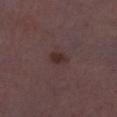The lesion was tiled from a total-body skin photograph and was not biopsied.
On the left lower leg.
A 15 mm close-up extracted from a 3D total-body photography capture.
A female subject in their 30s.